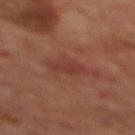biopsy_status: not biopsied; imaged during a skin examination
patient:
  sex: male
  age_approx: 70
site: mid back
lighting: cross-polarized
lesion_size:
  long_diameter_mm_approx: 3.0
image:
  source: total-body photography crop
  field_of_view_mm: 15